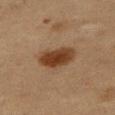Context:
A lesion tile, about 15 mm wide, cut from a 3D total-body photograph. A female patient roughly 40 years of age. On the right thigh.